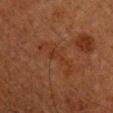Assessment:
The lesion was photographed on a routine skin check and not biopsied; there is no pathology result.
Background:
This is a cross-polarized tile. The recorded lesion diameter is about 5.5 mm. The subject is a male in their mid-70s. A 15 mm close-up extracted from a 3D total-body photography capture. Located on the right upper arm. Automated image analysis of the tile measured an area of roughly 6 mm², a shape eccentricity near 0.95, and two-axis asymmetry of about 0.65. The analysis additionally found a border-irregularity rating of about 9.5/10, a within-lesion color-variation index near 0.5/10, and radial color variation of about 0.